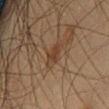<case>
<biopsy_status>not biopsied; imaged during a skin examination</biopsy_status>
<automated_metrics>
  <border_irregularity_0_10>4.5</border_irregularity_0_10>
  <peripheral_color_asymmetry>0.5</peripheral_color_asymmetry>
  <lesion_detection_confidence_0_100>90</lesion_detection_confidence_0_100>
</automated_metrics>
<lighting>cross-polarized</lighting>
<site>left thigh</site>
<lesion_size>
  <long_diameter_mm_approx>3.0</long_diameter_mm_approx>
</lesion_size>
<patient>
  <sex>male</sex>
  <age_approx>45</age_approx>
</patient>
<image>
  <source>total-body photography crop</source>
  <field_of_view_mm>15</field_of_view_mm>
</image>
</case>